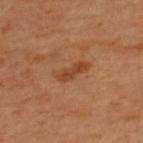biopsy_status: not biopsied; imaged during a skin examination
site: back
patient:
  sex: male
  age_approx: 50
image:
  source: total-body photography crop
  field_of_view_mm: 15
lighting: cross-polarized